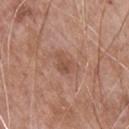Assessment:
Captured during whole-body skin photography for melanoma surveillance; the lesion was not biopsied.
Image and clinical context:
From the chest. Automated tile analysis of the lesion measured an automated nevus-likeness rating near 5 out of 100. A 15 mm crop from a total-body photograph taken for skin-cancer surveillance. The subject is a male approximately 65 years of age.